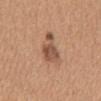Part of a total-body skin-imaging series; this lesion was reviewed on a skin check and was not flagged for biopsy. On the mid back. The subject is a female aged around 40. The lesion's longest dimension is about 6 mm. Cropped from a whole-body photographic skin survey; the tile spans about 15 mm. The lesion-visualizer software estimated a lesion color around L≈55 a*≈20 b*≈31 in CIELAB, a lesion–skin lightness drop of about 9, and a normalized lesion–skin contrast near 6.5. The software also gave a border-irregularity rating of about 4/10, a color-variation rating of about 5.5/10, and radial color variation of about 1.5. It also reported an automated nevus-likeness rating near 70 out of 100.